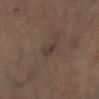Notes:
- follow-up · total-body-photography surveillance lesion; no biopsy
- automated lesion analysis · a lesion area of about 2.5 mm², an eccentricity of roughly 0.95, and two-axis asymmetry of about 0.25; about 7 CIELAB-L* units darker than the surrounding skin and a normalized border contrast of about 7; a within-lesion color-variation index near 0/10
- imaging modality · total-body-photography crop, ~15 mm field of view
- subject · female, aged approximately 70
- lesion size · ≈2.5 mm
- anatomic site · the left lower leg
- lighting · cross-polarized illumination